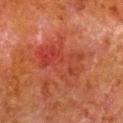Q: Was a biopsy performed?
A: imaged on a skin check; not biopsied
Q: What is the anatomic site?
A: the right lower leg
Q: Lesion size?
A: about 6.5 mm
Q: Patient demographics?
A: male, aged 78–82
Q: What is the imaging modality?
A: ~15 mm tile from a whole-body skin photo
Q: How was the tile lit?
A: cross-polarized
Q: What did automated image analysis measure?
A: a lesion color around L≈35 a*≈29 b*≈29 in CIELAB, about 6 CIELAB-L* units darker than the surrounding skin, and a normalized border contrast of about 5.5; border irregularity of about 4.5 on a 0–10 scale, internal color variation of about 7.5 on a 0–10 scale, and radial color variation of about 3